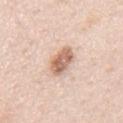Clinical impression: Part of a total-body skin-imaging series; this lesion was reviewed on a skin check and was not flagged for biopsy. Context: A 15 mm crop from a total-body photograph taken for skin-cancer surveillance. The subject is a male about 50 years old. The lesion is located on the back.